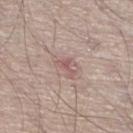notes: catalogued during a skin exam; not biopsied
acquisition: total-body-photography crop, ~15 mm field of view
illumination: white-light
patient: male, aged 53 to 57
location: the right lower leg
image-analysis metrics: an area of roughly 2.5 mm², an outline eccentricity of about 0.85 (0 = round, 1 = elongated), and a symmetry-axis asymmetry near 0.6; a border-irregularity rating of about 6/10, internal color variation of about 0 on a 0–10 scale, and a peripheral color-asymmetry measure near 0